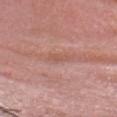Recorded during total-body skin imaging; not selected for excision or biopsy.
A 15 mm close-up extracted from a 3D total-body photography capture.
A male subject, about 60 years old.
From the head or neck.
The tile uses white-light illumination.
The recorded lesion diameter is about 3 mm.